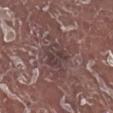The lesion was tiled from a total-body skin photograph and was not biopsied.
The tile uses white-light illumination.
A male subject, aged around 75.
Located on the right lower leg.
An algorithmic analysis of the crop reported a lesion area of about 7.5 mm² and a symmetry-axis asymmetry near 0.4. The analysis additionally found an average lesion color of about L≈38 a*≈16 b*≈16 (CIELAB), roughly 7 lightness units darker than nearby skin, and a normalized lesion–skin contrast near 6.5. The analysis additionally found a nevus-likeness score of about 0/100 and lesion-presence confidence of about 55/100.
A lesion tile, about 15 mm wide, cut from a 3D total-body photograph.
Measured at roughly 3.5 mm in maximum diameter.